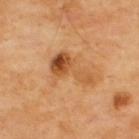The lesion was tiled from a total-body skin photograph and was not biopsied. A lesion tile, about 15 mm wide, cut from a 3D total-body photograph. Located on the upper back. A male patient in their 70s.